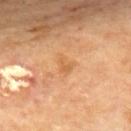Impression: The lesion was tiled from a total-body skin photograph and was not biopsied. Image and clinical context: Imaged with cross-polarized lighting. Automated image analysis of the tile measured an area of roughly 3.5 mm². The software also gave a nevus-likeness score of about 0/100 and a lesion-detection confidence of about 100/100. Located on the mid back. The patient is a male aged 68 to 72. Measured at roughly 2.5 mm in maximum diameter. A 15 mm close-up extracted from a 3D total-body photography capture.